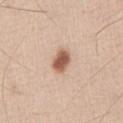automated lesion analysis: a lesion area of about 5.5 mm², an outline eccentricity of about 0.75 (0 = round, 1 = elongated), and two-axis asymmetry of about 0.15; an automated nevus-likeness rating near 100 out of 100 | body site: the chest | subject: male, in their mid-40s | lesion diameter: ~3 mm (longest diameter) | imaging modality: ~15 mm tile from a whole-body skin photo | lighting: white-light illumination.